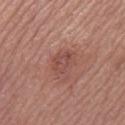Clinical impression:
Imaged during a routine full-body skin examination; the lesion was not biopsied and no histopathology is available.
Context:
Located on the leg. A female patient, about 50 years old. The total-body-photography lesion software estimated a mean CIELAB color near L≈48 a*≈24 b*≈25 and roughly 7 lightness units darker than nearby skin. And it measured a border-irregularity rating of about 2.5/10, a color-variation rating of about 3/10, and peripheral color asymmetry of about 1. A 15 mm close-up extracted from a 3D total-body photography capture.